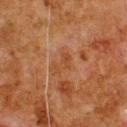Part of a total-body skin-imaging series; this lesion was reviewed on a skin check and was not flagged for biopsy.
Captured under cross-polarized illumination.
The lesion is on the upper back.
A male patient in their 80s.
The lesion's longest dimension is about 3 mm.
A 15 mm close-up tile from a total-body photography series done for melanoma screening.